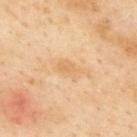{
  "biopsy_status": "not biopsied; imaged during a skin examination",
  "patient": {
    "sex": "male",
    "age_approx": 55
  },
  "lighting": "cross-polarized",
  "lesion_size": {
    "long_diameter_mm_approx": 3.0
  },
  "image": {
    "source": "total-body photography crop",
    "field_of_view_mm": 15
  },
  "automated_metrics": {
    "cielab_L": 70,
    "cielab_a": 19,
    "cielab_b": 42,
    "vs_skin_darker_L": 7.0,
    "border_irregularity_0_10": 3.0,
    "color_variation_0_10": 1.5,
    "peripheral_color_asymmetry": 0.5,
    "lesion_detection_confidence_0_100": 100
  },
  "site": "upper back"
}